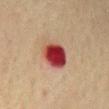Notes:
- biopsy status: total-body-photography surveillance lesion; no biopsy
- location: the mid back
- image-analysis metrics: a lesion color around L≈37 a*≈31 b*≈25 in CIELAB and a normalized border contrast of about 14; a classifier nevus-likeness of about 0/100
- patient: male, roughly 75 years of age
- tile lighting: cross-polarized
- imaging modality: ~15 mm tile from a whole-body skin photo
- lesion diameter: ~3.5 mm (longest diameter)